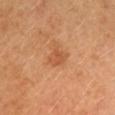  biopsy_status: not biopsied; imaged during a skin examination
  automated_metrics:
    vs_skin_darker_L: 7.0
    vs_skin_contrast_norm: 5.0
    border_irregularity_0_10: 3.0
    color_variation_0_10: 2.0
    peripheral_color_asymmetry: 0.5
  lighting: cross-polarized
  site: head or neck
  lesion_size:
    long_diameter_mm_approx: 3.0
  patient:
    sex: female
    age_approx: 40
  image:
    source: total-body photography crop
    field_of_view_mm: 15